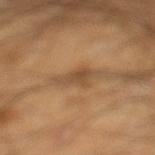biopsy status: catalogued during a skin exam; not biopsied
patient: male, in their 40s
lesion diameter: about 4 mm
location: the left lower leg
acquisition: 15 mm crop, total-body photography